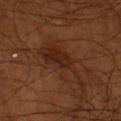workup = total-body-photography surveillance lesion; no biopsy
lesion diameter = ~8 mm (longest diameter)
subject = male, aged 68–72
site = the left forearm
automated metrics = peripheral color asymmetry of about 1; a classifier nevus-likeness of about 5/100 and a lesion-detection confidence of about 100/100
image source = total-body-photography crop, ~15 mm field of view